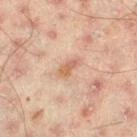| key | value |
|---|---|
| follow-up | catalogued during a skin exam; not biopsied |
| tile lighting | cross-polarized illumination |
| lesion size | about 2.5 mm |
| subject | male, about 45 years old |
| automated lesion analysis | a shape-asymmetry score of about 0.2 (0 = symmetric); an average lesion color of about L≈53 a*≈20 b*≈29 (CIELAB) and a normalized lesion–skin contrast near 7; a border-irregularity index near 2/10, a color-variation rating of about 0/10, and radial color variation of about 0; a nevus-likeness score of about 25/100 and lesion-presence confidence of about 100/100 |
| imaging modality | total-body-photography crop, ~15 mm field of view |
| anatomic site | the left thigh |